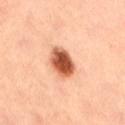A roughly 15 mm field-of-view crop from a total-body skin photograph. The tile uses cross-polarized illumination. A female patient, aged 33 to 37. The lesion's longest dimension is about 4 mm. The lesion is on the lower back.